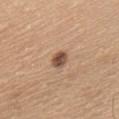workup: total-body-photography surveillance lesion; no biopsy
patient: female, in their mid- to late 50s
image source: total-body-photography crop, ~15 mm field of view
automated lesion analysis: a detector confidence of about 100 out of 100 that the crop contains a lesion
anatomic site: the chest